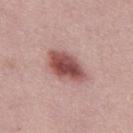Imaged during a routine full-body skin examination; the lesion was not biopsied and no histopathology is available.
A male patient aged around 30.
The tile uses white-light illumination.
The lesion's longest dimension is about 5 mm.
The lesion-visualizer software estimated about 16 CIELAB-L* units darker than the surrounding skin and a lesion-to-skin contrast of about 11 (normalized; higher = more distinct). The software also gave a border-irregularity rating of about 2/10 and a peripheral color-asymmetry measure near 1.5. It also reported a classifier nevus-likeness of about 95/100.
A roughly 15 mm field-of-view crop from a total-body skin photograph.